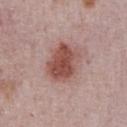Part of a total-body skin-imaging series; this lesion was reviewed on a skin check and was not flagged for biopsy.